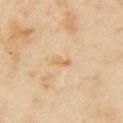Recorded during total-body skin imaging; not selected for excision or biopsy. The lesion-visualizer software estimated a lesion area of about 2.5 mm², an outline eccentricity of about 0.9 (0 = round, 1 = elongated), and a shape-asymmetry score of about 0.35 (0 = symmetric). The software also gave a lesion color around L≈65 a*≈16 b*≈39 in CIELAB, a lesion–skin lightness drop of about 7, and a normalized lesion–skin contrast near 5.5. It also reported a border-irregularity index near 3.5/10 and a within-lesion color-variation index near 0/10. It also reported a lesion-detection confidence of about 100/100. The lesion's longest dimension is about 2.5 mm. A female subject approximately 35 years of age. Located on the right upper arm. A 15 mm close-up extracted from a 3D total-body photography capture. Imaged with cross-polarized lighting.